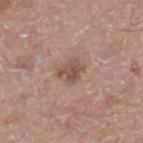Part of a total-body skin-imaging series; this lesion was reviewed on a skin check and was not flagged for biopsy. The lesion is located on the left thigh. The patient is a male aged 63 to 67. A 15 mm close-up extracted from a 3D total-body photography capture.